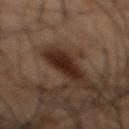Clinical impression: No biopsy was performed on this lesion — it was imaged during a full skin examination and was not determined to be concerning. Background: A male patient, about 50 years old. Cropped from a whole-body photographic skin survey; the tile spans about 15 mm. Imaged with cross-polarized lighting. From the back.